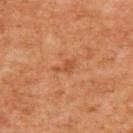Imaged during a routine full-body skin examination; the lesion was not biopsied and no histopathology is available. Longest diameter approximately 3 mm. Cropped from a whole-body photographic skin survey; the tile spans about 15 mm. A male subject aged approximately 60. On the front of the torso. The lesion-visualizer software estimated a lesion area of about 3 mm² and a shape eccentricity near 0.85. The analysis additionally found a border-irregularity rating of about 3.5/10, a within-lesion color-variation index near 1/10, and a peripheral color-asymmetry measure near 0.5. The tile uses cross-polarized illumination.